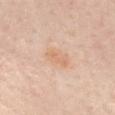Case summary:
– notes: catalogued during a skin exam; not biopsied
– location: the chest
– image source: total-body-photography crop, ~15 mm field of view
– patient: female, aged 48–52